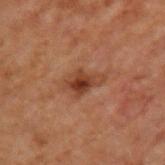Context:
Located on the upper back. This image is a 15 mm lesion crop taken from a total-body photograph. A male patient roughly 70 years of age.